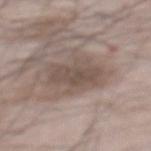notes: catalogued during a skin exam; not biopsied
TBP lesion metrics: a lesion area of about 14 mm², an outline eccentricity of about 0.65 (0 = round, 1 = elongated), and a symmetry-axis asymmetry near 0.2; a border-irregularity index near 3.5/10 and radial color variation of about 1
lesion size: ~5 mm (longest diameter)
imaging modality: total-body-photography crop, ~15 mm field of view
illumination: white-light illumination
location: the mid back
patient: male, aged approximately 65